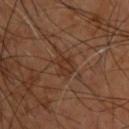workup: total-body-photography surveillance lesion; no biopsy
image source: 15 mm crop, total-body photography
illumination: cross-polarized illumination
body site: the upper back
subject: male, roughly 60 years of age
diameter: about 3.5 mm